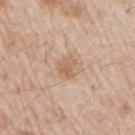  biopsy_status: not biopsied; imaged during a skin examination
  lighting: white-light
  lesion_size:
    long_diameter_mm_approx: 2.5
  image:
    source: total-body photography crop
    field_of_view_mm: 15
  patient:
    sex: male
    age_approx: 65
  site: right upper arm
  automated_metrics:
    cielab_L: 61
    cielab_a: 18
    cielab_b: 32
    vs_skin_darker_L: 8.0
    vs_skin_contrast_norm: 6.0
    border_irregularity_0_10: 3.5